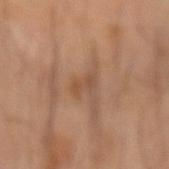Q: Was a biopsy performed?
A: no biopsy performed (imaged during a skin exam)
Q: Where on the body is the lesion?
A: the right forearm
Q: How was this image acquired?
A: total-body-photography crop, ~15 mm field of view
Q: Patient demographics?
A: male, aged 63–67